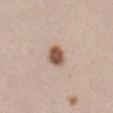follow-up=total-body-photography surveillance lesion; no biopsy | subject=female, in their 40s | anatomic site=the abdomen | acquisition=~15 mm crop, total-body skin-cancer survey | illumination=white-light.